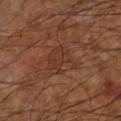The lesion was tiled from a total-body skin photograph and was not biopsied.
This is a cross-polarized tile.
A male patient, aged approximately 60.
The lesion is on the right lower leg.
This image is a 15 mm lesion crop taken from a total-body photograph.